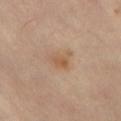Findings:
* workup — no biopsy performed (imaged during a skin exam)
* body site — the left thigh
* image source — ~15 mm crop, total-body skin-cancer survey
* automated lesion analysis — an area of roughly 4 mm², an outline eccentricity of about 0.55 (0 = round, 1 = elongated), and a shape-asymmetry score of about 0.55 (0 = symmetric); a mean CIELAB color near L≈56 a*≈18 b*≈33, about 7 CIELAB-L* units darker than the surrounding skin, and a normalized border contrast of about 6
* size — ≈3 mm
* patient — female, in their mid-40s